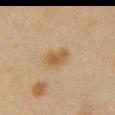Notes:
* workup: total-body-photography surveillance lesion; no biopsy
* lesion size: ~3.5 mm (longest diameter)
* illumination: cross-polarized illumination
* subject: female, approximately 40 years of age
* automated lesion analysis: an area of roughly 5.5 mm² and two-axis asymmetry of about 0.2; a lesion color around L≈46 a*≈13 b*≈31 in CIELAB and a lesion–skin lightness drop of about 8; a border-irregularity rating of about 2.5/10, internal color variation of about 2 on a 0–10 scale, and peripheral color asymmetry of about 0.5
* anatomic site: the front of the torso
* image source: ~15 mm tile from a whole-body skin photo